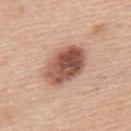Case summary:
• follow-up — total-body-photography surveillance lesion; no biopsy
• acquisition — ~15 mm crop, total-body skin-cancer survey
• tile lighting — white-light illumination
• patient — male, about 50 years old
• diameter — about 5.5 mm
• location — the upper back